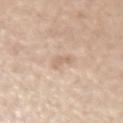{
  "biopsy_status": "not biopsied; imaged during a skin examination",
  "patient": {
    "sex": "male",
    "age_approx": 75
  },
  "site": "left upper arm",
  "lighting": "white-light",
  "image": {
    "source": "total-body photography crop",
    "field_of_view_mm": 15
  },
  "lesion_size": {
    "long_diameter_mm_approx": 3.0
  }
}